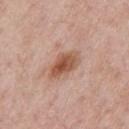Q: Was this lesion biopsied?
A: total-body-photography surveillance lesion; no biopsy
Q: Where on the body is the lesion?
A: the chest
Q: What are the patient's age and sex?
A: male, in their mid-50s
Q: What kind of image is this?
A: total-body-photography crop, ~15 mm field of view